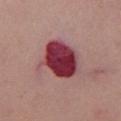<tbp_lesion>
<biopsy_status>not biopsied; imaged during a skin examination</biopsy_status>
</tbp_lesion>